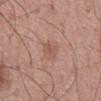This lesion was catalogued during total-body skin photography and was not selected for biopsy. A 15 mm close-up tile from a total-body photography series done for melanoma screening. Longest diameter approximately 3.5 mm. The subject is a male aged 53 to 57. The lesion is located on the back.